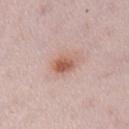The lesion was photographed on a routine skin check and not biopsied; there is no pathology result.
The lesion's longest dimension is about 3.5 mm.
A female patient, aged around 30.
The lesion is located on the left lower leg.
Cropped from a total-body skin-imaging series; the visible field is about 15 mm.
The total-body-photography lesion software estimated a footprint of about 5.5 mm² and an outline eccentricity of about 0.75 (0 = round, 1 = elongated). And it measured border irregularity of about 2.5 on a 0–10 scale, a color-variation rating of about 5/10, and radial color variation of about 1.5.
Imaged with white-light lighting.